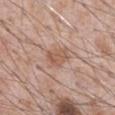Assessment:
The lesion was photographed on a routine skin check and not biopsied; there is no pathology result.
Clinical summary:
A close-up tile cropped from a whole-body skin photograph, about 15 mm across. A male subject, approximately 70 years of age. The recorded lesion diameter is about 3 mm. An algorithmic analysis of the crop reported roughly 8 lightness units darker than nearby skin. The analysis additionally found border irregularity of about 3 on a 0–10 scale and internal color variation of about 2.5 on a 0–10 scale. The analysis additionally found a classifier nevus-likeness of about 0/100 and lesion-presence confidence of about 100/100. Imaged with white-light lighting. The lesion is on the abdomen.